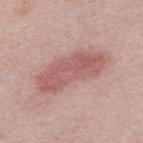{"biopsy_status": "not biopsied; imaged during a skin examination", "site": "upper back", "image": {"source": "total-body photography crop", "field_of_view_mm": 15}, "patient": {"sex": "male", "age_approx": 40}, "lesion_size": {"long_diameter_mm_approx": 8.0}, "automated_metrics": {"cielab_L": 57, "cielab_a": 24, "cielab_b": 23, "vs_skin_darker_L": 11.0, "vs_skin_contrast_norm": 7.0, "nevus_likeness_0_100": 55, "lesion_detection_confidence_0_100": 100}}